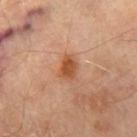biopsy_status: not biopsied; imaged during a skin examination
image:
  source: total-body photography crop
  field_of_view_mm: 15
lesion_size:
  long_diameter_mm_approx: 2.5
patient:
  sex: male
  age_approx: 70
site: right thigh
lighting: cross-polarized